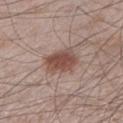notes: catalogued during a skin exam; not biopsied | anatomic site: the leg | acquisition: 15 mm crop, total-body photography | automated metrics: an area of roughly 9.5 mm²; a lesion color around L≈48 a*≈19 b*≈24 in CIELAB, roughly 12 lightness units darker than nearby skin, and a normalized lesion–skin contrast near 9; internal color variation of about 3.5 on a 0–10 scale and radial color variation of about 1; an automated nevus-likeness rating near 100 out of 100 and a detector confidence of about 100 out of 100 that the crop contains a lesion | subject: male, about 60 years old | diameter: ~4 mm (longest diameter) | illumination: white-light illumination.